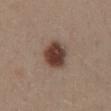Findings:
- notes · no biopsy performed (imaged during a skin exam)